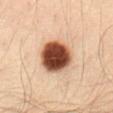• workup · catalogued during a skin exam; not biopsied
• body site · the abdomen
• patient · male, aged approximately 40
• size · ~4.5 mm (longest diameter)
• image-analysis metrics · a footprint of about 16 mm², an outline eccentricity of about 0.45 (0 = round, 1 = elongated), and a symmetry-axis asymmetry near 0.1; roughly 28 lightness units darker than nearby skin and a normalized border contrast of about 17; a border-irregularity index near 1/10 and peripheral color asymmetry of about 1.5
• acquisition · 15 mm crop, total-body photography
• illumination · cross-polarized